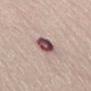Part of a total-body skin-imaging series; this lesion was reviewed on a skin check and was not flagged for biopsy.
A male subject aged 83 or older.
Imaged with white-light lighting.
Cropped from a total-body skin-imaging series; the visible field is about 15 mm.
On the lower back.